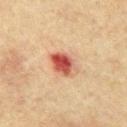Notes:
* biopsy status · total-body-photography surveillance lesion; no biopsy
* image source · ~15 mm tile from a whole-body skin photo
* patient · male, roughly 65 years of age
* location · the chest